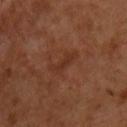{
  "biopsy_status": "not biopsied; imaged during a skin examination",
  "patient": {
    "sex": "male",
    "age_approx": 50
  },
  "image": {
    "source": "total-body photography crop",
    "field_of_view_mm": 15
  },
  "lesion_size": {
    "long_diameter_mm_approx": 3.5
  },
  "site": "chest"
}